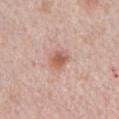{
  "biopsy_status": "not biopsied; imaged during a skin examination",
  "automated_metrics": {
    "area_mm2_approx": 5.5,
    "eccentricity": 0.55,
    "shape_asymmetry": 0.2,
    "cielab_L": 59,
    "cielab_a": 23,
    "cielab_b": 29,
    "vs_skin_darker_L": 10.0,
    "vs_skin_contrast_norm": 7.5,
    "nevus_likeness_0_100": 80
  },
  "lighting": "white-light",
  "patient": {
    "sex": "male",
    "age_approx": 70
  },
  "image": {
    "source": "total-body photography crop",
    "field_of_view_mm": 15
  },
  "lesion_size": {
    "long_diameter_mm_approx": 3.0
  },
  "site": "front of the torso"
}